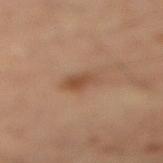The lesion was photographed on a routine skin check and not biopsied; there is no pathology result. On the left leg. This is a cross-polarized tile. The patient is a male about 50 years old. Longest diameter approximately 3.5 mm. Automated image analysis of the tile measured a lesion area of about 5.5 mm². The analysis additionally found a lesion color around L≈48 a*≈20 b*≈31 in CIELAB, a lesion–skin lightness drop of about 9, and a normalized lesion–skin contrast near 6.5. A 15 mm close-up tile from a total-body photography series done for melanoma screening.